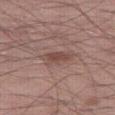Notes:
* workup · catalogued during a skin exam; not biopsied